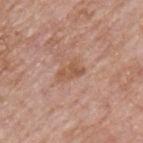| feature | finding |
|---|---|
| notes | no biopsy performed (imaged during a skin exam) |
| TBP lesion metrics | an area of roughly 5 mm², a shape eccentricity near 0.8, and a symmetry-axis asymmetry near 0.35; a lesion color around L≈55 a*≈22 b*≈32 in CIELAB, roughly 8 lightness units darker than nearby skin, and a lesion-to-skin contrast of about 6.5 (normalized; higher = more distinct); border irregularity of about 3.5 on a 0–10 scale and a within-lesion color-variation index near 2.5/10 |
| image source | ~15 mm tile from a whole-body skin photo |
| anatomic site | the front of the torso |
| diameter | ~3 mm (longest diameter) |
| patient | male, aged 73 to 77 |